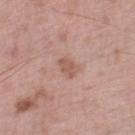patient — male, in their 70s
body site — the right lower leg
image — total-body-photography crop, ~15 mm field of view